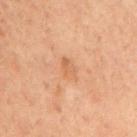Assessment: No biopsy was performed on this lesion — it was imaged during a full skin examination and was not determined to be concerning. Background: A male patient in their 60s. The recorded lesion diameter is about 2.5 mm. The lesion-visualizer software estimated an area of roughly 3.5 mm², a shape eccentricity near 0.7, and two-axis asymmetry of about 0.25. The analysis additionally found an average lesion color of about L≈48 a*≈19 b*≈30 (CIELAB), about 5 CIELAB-L* units darker than the surrounding skin, and a lesion-to-skin contrast of about 5 (normalized; higher = more distinct). And it measured border irregularity of about 2.5 on a 0–10 scale, a color-variation rating of about 2/10, and a peripheral color-asymmetry measure near 0.5. The lesion is located on the left upper arm. Captured under cross-polarized illumination. This image is a 15 mm lesion crop taken from a total-body photograph.